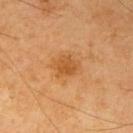biopsy status: total-body-photography surveillance lesion; no biopsy
tile lighting: cross-polarized
imaging modality: 15 mm crop, total-body photography
lesion diameter: ~3 mm (longest diameter)
TBP lesion metrics: a lesion area of about 5.5 mm²; a nevus-likeness score of about 60/100 and a lesion-detection confidence of about 100/100
patient: male, aged around 70
body site: the right upper arm